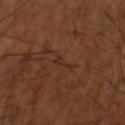Q: Was this lesion biopsied?
A: total-body-photography surveillance lesion; no biopsy
Q: What are the patient's age and sex?
A: male, aged around 65
Q: What lighting was used for the tile?
A: cross-polarized illumination
Q: What is the anatomic site?
A: the left lower leg
Q: How was this image acquired?
A: total-body-photography crop, ~15 mm field of view
Q: What did automated image analysis measure?
A: a footprint of about 3 mm², an eccentricity of roughly 0.95, and a symmetry-axis asymmetry near 0.65; a border-irregularity rating of about 7.5/10, internal color variation of about 0 on a 0–10 scale, and a peripheral color-asymmetry measure near 0; a detector confidence of about 70 out of 100 that the crop contains a lesion
Q: How large is the lesion?
A: about 3.5 mm